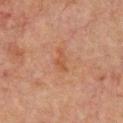No biopsy was performed on this lesion — it was imaged during a full skin examination and was not determined to be concerning.
A roughly 15 mm field-of-view crop from a total-body skin photograph.
Located on the upper back.
A male patient aged 63–67.